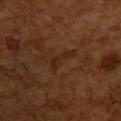The lesion was photographed on a routine skin check and not biopsied; there is no pathology result. A 15 mm crop from a total-body photograph taken for skin-cancer surveillance. The lesion-visualizer software estimated a shape eccentricity near 0.95 and a shape-asymmetry score of about 0.55 (0 = symmetric). The analysis additionally found a lesion color around L≈26 a*≈20 b*≈29 in CIELAB, about 5 CIELAB-L* units darker than the surrounding skin, and a normalized border contrast of about 5.5. The software also gave a within-lesion color-variation index near 0.5/10 and radial color variation of about 0. A female subject aged 53 to 57. The lesion is on the upper back. The tile uses cross-polarized illumination.